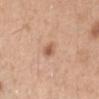A 15 mm crop from a total-body photograph taken for skin-cancer surveillance.
The subject is a male aged around 30.
Located on the right forearm.
An algorithmic analysis of the crop reported a lesion color around L≈58 a*≈22 b*≈33 in CIELAB, a lesion–skin lightness drop of about 10, and a lesion-to-skin contrast of about 7 (normalized; higher = more distinct). The software also gave a border-irregularity rating of about 2/10, a color-variation rating of about 2.5/10, and peripheral color asymmetry of about 1.
Longest diameter approximately 2.5 mm.
Captured under white-light illumination.